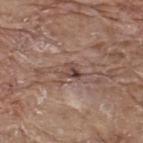workup: no biopsy performed (imaged during a skin exam)
image source: ~15 mm tile from a whole-body skin photo
anatomic site: the back
diameter: ≈2.5 mm
tile lighting: white-light illumination
subject: male, aged 68–72
image-analysis metrics: an area of roughly 3.5 mm², an outline eccentricity of about 0.65 (0 = round, 1 = elongated), and two-axis asymmetry of about 0.5; radial color variation of about 2; an automated nevus-likeness rating near 0 out of 100 and lesion-presence confidence of about 95/100